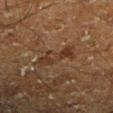Impression:
Part of a total-body skin-imaging series; this lesion was reviewed on a skin check and was not flagged for biopsy.
Background:
Cropped from a whole-body photographic skin survey; the tile spans about 15 mm. A male patient approximately 60 years of age. Located on the left lower leg.